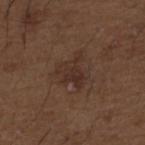workup=total-body-photography surveillance lesion; no biopsy | image source=15 mm crop, total-body photography | anatomic site=the upper back | subject=male, in their 50s | lesion diameter=≈4 mm.